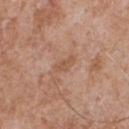* workup — no biopsy performed (imaged during a skin exam)
* lesion diameter — ~3 mm (longest diameter)
* site — the chest
* image-analysis metrics — an outline eccentricity of about 0.9 (0 = round, 1 = elongated) and a symmetry-axis asymmetry near 0.25; an average lesion color of about L≈54 a*≈21 b*≈31 (CIELAB) and a normalized border contrast of about 5.5
* image — 15 mm crop, total-body photography
* patient — male, in their mid- to late 60s
* illumination — white-light